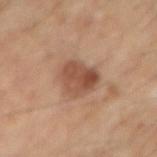This lesion was catalogued during total-body skin photography and was not selected for biopsy. The patient is a male aged 53–57. The recorded lesion diameter is about 4 mm. On the right forearm. An algorithmic analysis of the crop reported an average lesion color of about L≈51 a*≈21 b*≈31 (CIELAB), about 11 CIELAB-L* units darker than the surrounding skin, and a normalized lesion–skin contrast near 8. The software also gave border irregularity of about 2.5 on a 0–10 scale and a color-variation rating of about 5.5/10. The software also gave a nevus-likeness score of about 75/100 and a lesion-detection confidence of about 100/100. Cropped from a whole-body photographic skin survey; the tile spans about 15 mm.